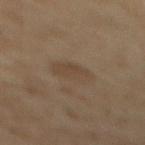Recorded during total-body skin imaging; not selected for excision or biopsy. Located on the mid back. A female subject aged 58–62. A 15 mm close-up tile from a total-body photography series done for melanoma screening.